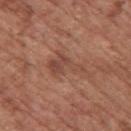follow-up=catalogued during a skin exam; not biopsied
lighting=white-light illumination
automated metrics=an area of roughly 8 mm², an eccentricity of roughly 0.85, and two-axis asymmetry of about 0.6; a mean CIELAB color near L≈46 a*≈22 b*≈28; a border-irregularity rating of about 8.5/10, internal color variation of about 4 on a 0–10 scale, and radial color variation of about 1.5
image=~15 mm tile from a whole-body skin photo
location=the upper back
diameter=~5 mm (longest diameter)
subject=male, aged around 75